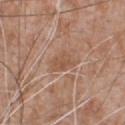biopsy status: catalogued during a skin exam; not biopsied | image source: 15 mm crop, total-body photography | patient: male, aged 63 to 67 | site: the chest | tile lighting: white-light.